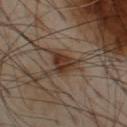On the chest. About 3.5 mm across. Automated image analysis of the tile measured a mean CIELAB color near L≈34 a*≈16 b*≈26 and roughly 11 lightness units darker than nearby skin. And it measured an automated nevus-likeness rating near 85 out of 100 and a lesion-detection confidence of about 100/100. A male subject, about 55 years old. Cropped from a whole-body photographic skin survey; the tile spans about 15 mm.